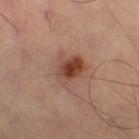follow-up: catalogued during a skin exam; not biopsied | automated lesion analysis: a footprint of about 9.5 mm², a shape eccentricity near 0.45, and two-axis asymmetry of about 0.3; internal color variation of about 7.5 on a 0–10 scale and a peripheral color-asymmetry measure near 2; an automated nevus-likeness rating near 95 out of 100 | body site: the right thigh | lesion size: ~3.5 mm (longest diameter) | imaging modality: ~15 mm tile from a whole-body skin photo | illumination: cross-polarized illumination.